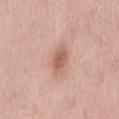workup: imaged on a skin check; not biopsied | body site: the back | tile lighting: white-light illumination | subject: male, aged around 55 | acquisition: ~15 mm tile from a whole-body skin photo | diameter: ≈3 mm.